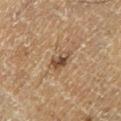workup = no biopsy performed (imaged during a skin exam) | image source = ~15 mm crop, total-body skin-cancer survey | location = the left lower leg | subject = male, about 65 years old.